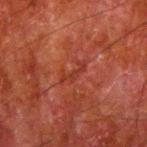Clinical impression:
The lesion was tiled from a total-body skin photograph and was not biopsied.
Clinical summary:
Located on the arm. A region of skin cropped from a whole-body photographic capture, roughly 15 mm wide. The patient is a male approximately 80 years of age.